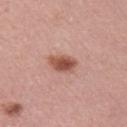Captured during whole-body skin photography for melanoma surveillance; the lesion was not biopsied. The recorded lesion diameter is about 3 mm. Located on the right upper arm. A female patient about 50 years old. A close-up tile cropped from a whole-body skin photograph, about 15 mm across.